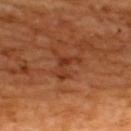Findings:
• notes — no biopsy performed (imaged during a skin exam)
• anatomic site — the back
• imaging modality — ~15 mm crop, total-body skin-cancer survey
• subject — male, aged approximately 65